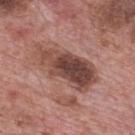– biopsy status — total-body-photography surveillance lesion; no biopsy
– imaging modality — ~15 mm crop, total-body skin-cancer survey
– lighting — white-light illumination
– location — the mid back
– patient — male, about 70 years old